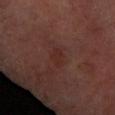<lesion>
<biopsy_status>not biopsied; imaged during a skin examination</biopsy_status>
<image>
  <source>total-body photography crop</source>
  <field_of_view_mm>15</field_of_view_mm>
</image>
<lesion_size>
  <long_diameter_mm_approx>2.5</long_diameter_mm_approx>
</lesion_size>
<patient>
  <sex>female</sex>
  <age_approx>65</age_approx>
</patient>
<site>left forearm</site>
<automated_metrics>
  <area_mm2_approx>3.5</area_mm2_approx>
  <cielab_L>26</cielab_L>
  <cielab_a>21</cielab_a>
  <cielab_b>21</cielab_b>
  <vs_skin_darker_L>4.0</vs_skin_darker_L>
  <vs_skin_contrast_norm>5.0</vs_skin_contrast_norm>
  <border_irregularity_0_10>2.5</border_irregularity_0_10>
  <color_variation_0_10>1.0</color_variation_0_10>
</automated_metrics>
<lighting>cross-polarized</lighting>
</lesion>